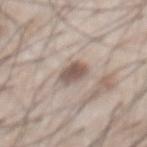Clinical summary:
The lesion-visualizer software estimated a shape eccentricity near 0.6 and a symmetry-axis asymmetry near 0.2. The analysis additionally found border irregularity of about 2 on a 0–10 scale, a color-variation rating of about 3/10, and a peripheral color-asymmetry measure near 1. And it measured a classifier nevus-likeness of about 85/100 and lesion-presence confidence of about 75/100. The lesion is located on the front of the torso. Cropped from a total-body skin-imaging series; the visible field is about 15 mm. A male subject in their 60s. About 3 mm across.